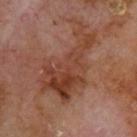A 15 mm crop from a total-body photograph taken for skin-cancer surveillance. The tile uses cross-polarized illumination. Located on the upper back. The patient is a male approximately 70 years of age.